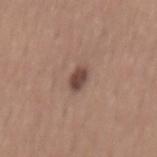Notes:
- biopsy status · imaged on a skin check; not biopsied
- patient · male, aged approximately 55
- imaging modality · ~15 mm tile from a whole-body skin photo
- anatomic site · the mid back